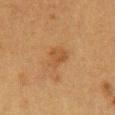Imaged during a routine full-body skin examination; the lesion was not biopsied and no histopathology is available. A close-up tile cropped from a whole-body skin photograph, about 15 mm across. The tile uses cross-polarized illumination. The patient is a female approximately 40 years of age. On the chest. Automated tile analysis of the lesion measured a lesion area of about 5.5 mm² and an eccentricity of roughly 0.7. The analysis additionally found an average lesion color of about L≈42 a*≈18 b*≈33 (CIELAB) and a normalized lesion–skin contrast near 5. It also reported border irregularity of about 3.5 on a 0–10 scale and a peripheral color-asymmetry measure near 0.5.